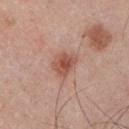Imaged during a routine full-body skin examination; the lesion was not biopsied and no histopathology is available. An algorithmic analysis of the crop reported an area of roughly 6.5 mm². The analysis additionally found a peripheral color-asymmetry measure near 1. And it measured a classifier nevus-likeness of about 90/100 and a detector confidence of about 100 out of 100 that the crop contains a lesion. The lesion is on the chest. A male patient in their mid- to late 20s. This is a white-light tile. A region of skin cropped from a whole-body photographic capture, roughly 15 mm wide.